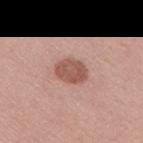{"biopsy_status": "not biopsied; imaged during a skin examination", "lighting": "white-light", "patient": {"sex": "male", "age_approx": 40}, "image": {"source": "total-body photography crop", "field_of_view_mm": 15}, "site": "right upper arm", "lesion_size": {"long_diameter_mm_approx": 3.5}}